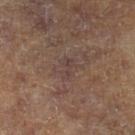| feature | finding |
|---|---|
| anatomic site | the left lower leg |
| acquisition | ~15 mm tile from a whole-body skin photo |
| automated lesion analysis | an area of roughly 3.5 mm² and a shape eccentricity near 0.8; a classifier nevus-likeness of about 0/100 and a lesion-detection confidence of about 50/100 |
| illumination | cross-polarized illumination |
| lesion diameter | ≈2.5 mm |
| subject | male, aged 83 or older |